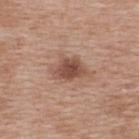Case summary:
* workup · no biopsy performed (imaged during a skin exam)
* image source · ~15 mm crop, total-body skin-cancer survey
* location · the upper back
* subject · female, in their 40s